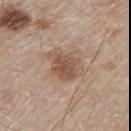Part of a total-body skin-imaging series; this lesion was reviewed on a skin check and was not flagged for biopsy. The subject is a male aged 78–82. The lesion is on the back. A 15 mm close-up tile from a total-body photography series done for melanoma screening. Longest diameter approximately 4.5 mm.